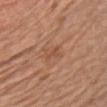No biopsy was performed on this lesion — it was imaged during a full skin examination and was not determined to be concerning. The patient is a male aged around 80. A 15 mm close-up extracted from a 3D total-body photography capture. Located on the front of the torso.